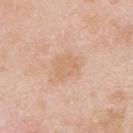follow-up — total-body-photography surveillance lesion; no biopsy | site — the upper back | TBP lesion metrics — an area of roughly 6 mm², an eccentricity of roughly 0.55, and a symmetry-axis asymmetry near 0.25; internal color variation of about 2 on a 0–10 scale and radial color variation of about 0.5; a lesion-detection confidence of about 100/100 | imaging modality — ~15 mm tile from a whole-body skin photo | patient — male, roughly 25 years of age | tile lighting — white-light.